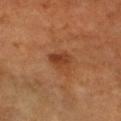workup = total-body-photography surveillance lesion; no biopsy | subject = male, roughly 60 years of age | acquisition = 15 mm crop, total-body photography | site = the head or neck.